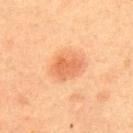Imaged during a routine full-body skin examination; the lesion was not biopsied and no histopathology is available. The lesion's longest dimension is about 4 mm. The tile uses cross-polarized illumination. The subject is a male about 60 years old. This image is a 15 mm lesion crop taken from a total-body photograph. The lesion is on the upper back.